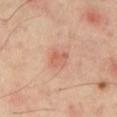<case>
<biopsy_status>not biopsied; imaged during a skin examination</biopsy_status>
<image>
  <source>total-body photography crop</source>
  <field_of_view_mm>15</field_of_view_mm>
</image>
<site>right thigh</site>
<lighting>cross-polarized</lighting>
</case>